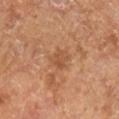Q: Was a biopsy performed?
A: catalogued during a skin exam; not biopsied
Q: Illumination type?
A: cross-polarized
Q: What did automated image analysis measure?
A: a lesion area of about 4.5 mm² and an eccentricity of roughly 0.55; a mean CIELAB color near L≈50 a*≈22 b*≈34 and a lesion–skin lightness drop of about 7; a border-irregularity rating of about 3/10, a color-variation rating of about 2/10, and peripheral color asymmetry of about 0.5; an automated nevus-likeness rating near 0 out of 100 and lesion-presence confidence of about 100/100
Q: What is the imaging modality?
A: ~15 mm tile from a whole-body skin photo
Q: Who is the patient?
A: male, roughly 65 years of age
Q: Lesion size?
A: ~2.5 mm (longest diameter)